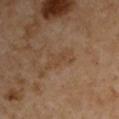workup — no biopsy performed (imaged during a skin exam)
lighting — cross-polarized
location — the left upper arm
acquisition — ~15 mm crop, total-body skin-cancer survey
automated lesion analysis — a lesion color around L≈44 a*≈17 b*≈30 in CIELAB, roughly 5 lightness units darker than nearby skin, and a normalized border contrast of about 4.5; a nevus-likeness score of about 0/100 and a detector confidence of about 100 out of 100 that the crop contains a lesion
size — ≈3.5 mm
subject — male, approximately 55 years of age